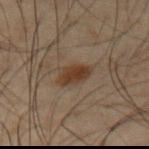A male patient roughly 50 years of age.
The total-body-photography lesion software estimated a lesion area of about 5.5 mm² and a shape-asymmetry score of about 0.25 (0 = symmetric). The software also gave a border-irregularity rating of about 2/10, a color-variation rating of about 2/10, and radial color variation of about 0.5.
Located on the left upper arm.
About 3.5 mm across.
A lesion tile, about 15 mm wide, cut from a 3D total-body photograph.
This is a cross-polarized tile.